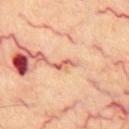| field | value |
|---|---|
| workup | catalogued during a skin exam; not biopsied |
| tile lighting | cross-polarized |
| subject | aged 63–67 |
| body site | the chest |
| imaging modality | total-body-photography crop, ~15 mm field of view |
| lesion diameter | ≈3 mm |